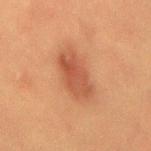Assessment: The lesion was tiled from a total-body skin photograph and was not biopsied. Context: A 15 mm close-up tile from a total-body photography series done for melanoma screening. Imaged with cross-polarized lighting. From the mid back. A female patient aged around 55. An algorithmic analysis of the crop reported a lesion area of about 13 mm², a shape eccentricity near 0.85, and two-axis asymmetry of about 0.15. The software also gave border irregularity of about 1.5 on a 0–10 scale and radial color variation of about 1.